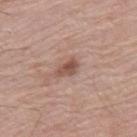<lesion>
  <biopsy_status>not biopsied; imaged during a skin examination</biopsy_status>
  <site>right thigh</site>
  <automated_metrics>
    <eccentricity>0.8</eccentricity>
    <shape_asymmetry>0.3</shape_asymmetry>
    <cielab_L>51</cielab_L>
    <cielab_a>20</cielab_a>
    <cielab_b>26</cielab_b>
    <vs_skin_darker_L>11.0</vs_skin_darker_L>
    <border_irregularity_0_10>3.0</border_irregularity_0_10>
    <peripheral_color_asymmetry>1.5</peripheral_color_asymmetry>
    <nevus_likeness_0_100>70</nevus_likeness_0_100>
    <lesion_detection_confidence_0_100>100</lesion_detection_confidence_0_100>
  </automated_metrics>
  <lesion_size>
    <long_diameter_mm_approx>3.0</long_diameter_mm_approx>
  </lesion_size>
  <patient>
    <sex>male</sex>
    <age_approx>80</age_approx>
  </patient>
  <lighting>white-light</lighting>
  <image>
    <source>total-body photography crop</source>
    <field_of_view_mm>15</field_of_view_mm>
  </image>
</lesion>